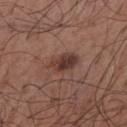<record>
<automated_metrics>
  <color_variation_0_10>5.0</color_variation_0_10>
  <peripheral_color_asymmetry>1.5</peripheral_color_asymmetry>
</automated_metrics>
<site>arm</site>
<lighting>white-light</lighting>
<image>
  <source>total-body photography crop</source>
  <field_of_view_mm>15</field_of_view_mm>
</image>
<patient>
  <sex>male</sex>
  <age_approx>65</age_approx>
</patient>
</record>